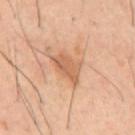Assessment: No biopsy was performed on this lesion — it was imaged during a full skin examination and was not determined to be concerning. Clinical summary: Located on the back. The tile uses cross-polarized illumination. Cropped from a total-body skin-imaging series; the visible field is about 15 mm. A male subject about 40 years old.